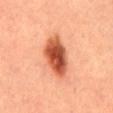follow-up: total-body-photography surveillance lesion; no biopsy | lesion diameter: ~5.5 mm (longest diameter) | image: total-body-photography crop, ~15 mm field of view | image-analysis metrics: an area of roughly 14 mm², a shape eccentricity near 0.8, and a shape-asymmetry score of about 0.15 (0 = symmetric); a lesion color around L≈51 a*≈30 b*≈36 in CIELAB, a lesion–skin lightness drop of about 18, and a lesion-to-skin contrast of about 11.5 (normalized; higher = more distinct) | patient: female, aged around 30 | location: the abdomen | tile lighting: cross-polarized illumination.